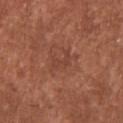Impression: Captured during whole-body skin photography for melanoma surveillance; the lesion was not biopsied. Clinical summary: Captured under white-light illumination. Cropped from a total-body skin-imaging series; the visible field is about 15 mm. The lesion is located on the back. Measured at roughly 2.5 mm in maximum diameter. The patient is a male about 45 years old.